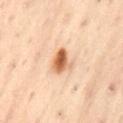Case summary:
- follow-up: no biopsy performed (imaged during a skin exam)
- body site: the back
- illumination: cross-polarized illumination
- patient: male, in their mid-50s
- diameter: ~3.5 mm (longest diameter)
- acquisition: total-body-photography crop, ~15 mm field of view